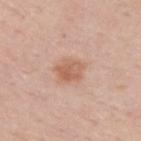Assessment: Captured during whole-body skin photography for melanoma surveillance; the lesion was not biopsied. Background: This image is a 15 mm lesion crop taken from a total-body photograph. Captured under white-light illumination. From the upper back. An algorithmic analysis of the crop reported an outline eccentricity of about 0.6 (0 = round, 1 = elongated) and two-axis asymmetry of about 0.2. And it measured a lesion color around L≈60 a*≈22 b*≈31 in CIELAB. It also reported a nevus-likeness score of about 60/100 and a lesion-detection confidence of about 100/100. The subject is a male aged 58 to 62.